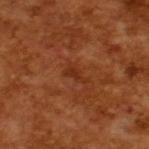{"biopsy_status": "not biopsied; imaged during a skin examination", "lighting": "cross-polarized", "image": {"source": "total-body photography crop", "field_of_view_mm": 15}, "patient": {"sex": "male", "age_approx": 65}, "lesion_size": {"long_diameter_mm_approx": 3.0}}